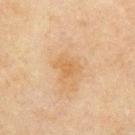Assessment: The lesion was photographed on a routine skin check and not biopsied; there is no pathology result. Acquisition and patient details: The lesion is located on the chest. Automated image analysis of the tile measured a border-irregularity rating of about 3.5/10, internal color variation of about 2 on a 0–10 scale, and a peripheral color-asymmetry measure near 0.5. A lesion tile, about 15 mm wide, cut from a 3D total-body photograph. Captured under cross-polarized illumination. About 3 mm across. A male patient about 70 years old.